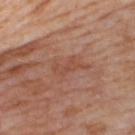  site: right thigh
  patient:
    sex: female
    age_approx: 55
  image:
    source: total-body photography crop
    field_of_view_mm: 15
  lighting: cross-polarized